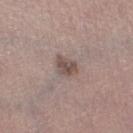Background: About 2.5 mm across. Cropped from a whole-body photographic skin survey; the tile spans about 15 mm. Imaged with white-light lighting. A female subject, in their mid- to late 60s. The lesion is located on the leg.